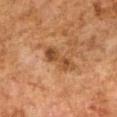{
  "biopsy_status": "not biopsied; imaged during a skin examination",
  "site": "right upper arm",
  "patient": {
    "sex": "female",
    "age_approx": 60
  },
  "image": {
    "source": "total-body photography crop",
    "field_of_view_mm": 15
  }
}